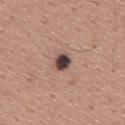Findings:
– notes: total-body-photography surveillance lesion; no biopsy
– image: ~15 mm crop, total-body skin-cancer survey
– automated lesion analysis: a mean CIELAB color near L≈43 a*≈16 b*≈18, a lesion–skin lightness drop of about 19, and a lesion-to-skin contrast of about 14.5 (normalized; higher = more distinct)
– body site: the mid back
– lesion size: ~2.5 mm (longest diameter)
– subject: male, in their mid- to late 40s